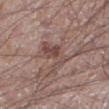location — the left lower leg | imaging modality — ~15 mm tile from a whole-body skin photo | automated metrics — an average lesion color of about L≈46 a*≈17 b*≈21 (CIELAB) and a lesion-to-skin contrast of about 6.5 (normalized; higher = more distinct); a classifier nevus-likeness of about 15/100 | tile lighting — white-light | subject — male, aged around 60 | size — ≈4 mm.